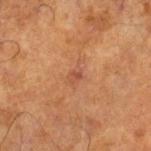image source: total-body-photography crop, ~15 mm field of view; patient: male, aged 68–72; illumination: cross-polarized; lesion size: ≈3 mm; site: the right lower leg.